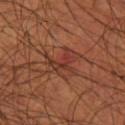  biopsy_status: not biopsied; imaged during a skin examination
  automated_metrics:
    cielab_L: 34
    cielab_a: 25
    cielab_b: 27
    vs_skin_darker_L: 7.0
    vs_skin_contrast_norm: 6.5
    border_irregularity_0_10: 8.5
    peripheral_color_asymmetry: 0.0
    nevus_likeness_0_100: 5
    lesion_detection_confidence_0_100: 90
  patient:
    sex: male
    age_approx: 70
  image:
    source: total-body photography crop
    field_of_view_mm: 15
  site: right thigh
  lighting: cross-polarized